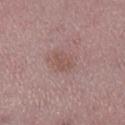The lesion was tiled from a total-body skin photograph and was not biopsied. Cropped from a whole-body photographic skin survey; the tile spans about 15 mm. The lesion is located on the left lower leg. A female subject, in their mid- to late 40s.